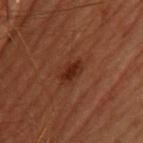{
  "biopsy_status": "not biopsied; imaged during a skin examination",
  "automated_metrics": {
    "area_mm2_approx": 4.5,
    "eccentricity": 0.8,
    "shape_asymmetry": 0.25,
    "cielab_L": 24,
    "cielab_a": 22,
    "cielab_b": 26,
    "vs_skin_darker_L": 7.0,
    "vs_skin_contrast_norm": 8.0,
    "border_irregularity_0_10": 2.5,
    "color_variation_0_10": 2.5,
    "peripheral_color_asymmetry": 1.0
  },
  "image": {
    "source": "total-body photography crop",
    "field_of_view_mm": 15
  },
  "site": "upper back",
  "lesion_size": {
    "long_diameter_mm_approx": 3.0
  },
  "lighting": "cross-polarized",
  "patient": {
    "sex": "female",
    "age_approx": 50
  }
}